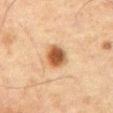Assessment: No biopsy was performed on this lesion — it was imaged during a full skin examination and was not determined to be concerning. Clinical summary: The total-body-photography lesion software estimated about 14 CIELAB-L* units darker than the surrounding skin and a normalized border contrast of about 11. It also reported a border-irregularity index near 1/10, a color-variation rating of about 3.5/10, and a peripheral color-asymmetry measure near 1. And it measured an automated nevus-likeness rating near 100 out of 100 and a detector confidence of about 100 out of 100 that the crop contains a lesion. On the abdomen. A male patient in their mid-70s. A roughly 15 mm field-of-view crop from a total-body skin photograph. Imaged with cross-polarized lighting.